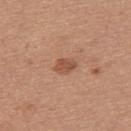{"biopsy_status": "not biopsied; imaged during a skin examination", "patient": {"sex": "female", "age_approx": 35}, "automated_metrics": {"area_mm2_approx": 4.5, "eccentricity": 0.7, "shape_asymmetry": 0.25}, "image": {"source": "total-body photography crop", "field_of_view_mm": 15}, "site": "upper back", "lighting": "white-light"}